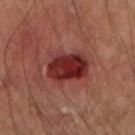No biopsy was performed on this lesion — it was imaged during a full skin examination and was not determined to be concerning. Imaged with cross-polarized lighting. Located on the right forearm. The recorded lesion diameter is about 4.5 mm. A lesion tile, about 15 mm wide, cut from a 3D total-body photograph. The subject is in their mid-50s. The total-body-photography lesion software estimated a lesion color around L≈31 a*≈30 b*≈24 in CIELAB, a lesion–skin lightness drop of about 14, and a normalized border contrast of about 12. It also reported a border-irregularity rating of about 2/10 and a peripheral color-asymmetry measure near 1.5.